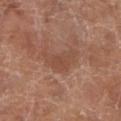| feature | finding |
|---|---|
| workup | total-body-photography surveillance lesion; no biopsy |
| lighting | white-light illumination |
| image-analysis metrics | a footprint of about 7.5 mm² and a shape eccentricity near 0.9; a classifier nevus-likeness of about 0/100 and lesion-presence confidence of about 100/100 |
| subject | female, in their 80s |
| lesion diameter | ~5 mm (longest diameter) |
| anatomic site | the left lower leg |
| image source | ~15 mm crop, total-body skin-cancer survey |